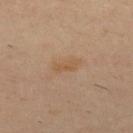The lesion was photographed on a routine skin check and not biopsied; there is no pathology result. The tile uses cross-polarized illumination. The recorded lesion diameter is about 3 mm. The lesion is located on the upper back. A male subject, approximately 40 years of age. A roughly 15 mm field-of-view crop from a total-body skin photograph. The total-body-photography lesion software estimated a lesion area of about 4 mm², an outline eccentricity of about 0.9 (0 = round, 1 = elongated), and a symmetry-axis asymmetry near 0.25. And it measured a color-variation rating of about 1.5/10 and radial color variation of about 0.5. The software also gave an automated nevus-likeness rating near 5 out of 100 and lesion-presence confidence of about 100/100.